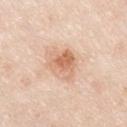  lesion_size:
    long_diameter_mm_approx: 4.0
  site: right upper arm
  image:
    source: total-body photography crop
    field_of_view_mm: 15
  patient:
    sex: male
    age_approx: 35
  lighting: white-light